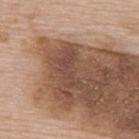| field | value |
|---|---|
| workup | no biopsy performed (imaged during a skin exam) |
| automated metrics | a lesion area of about 5.5 mm²; a mean CIELAB color near L≈45 a*≈18 b*≈26, a lesion–skin lightness drop of about 7, and a lesion-to-skin contrast of about 6 (normalized; higher = more distinct) |
| lesion size | ≈4.5 mm |
| site | the upper back |
| imaging modality | 15 mm crop, total-body photography |
| patient | male, in their mid-70s |
| lighting | white-light |